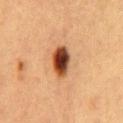No biopsy was performed on this lesion — it was imaged during a full skin examination and was not determined to be concerning.
A female patient, about 40 years old.
Located on the chest.
A 15 mm close-up extracted from a 3D total-body photography capture.
Imaged with cross-polarized lighting.